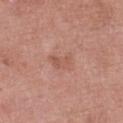notes — total-body-photography surveillance lesion; no biopsy | subject — female, roughly 70 years of age | image source — ~15 mm crop, total-body skin-cancer survey | automated metrics — a mean CIELAB color near L≈55 a*≈23 b*≈28, about 7 CIELAB-L* units darker than the surrounding skin, and a normalized border contrast of about 5 | lighting — white-light | lesion size — ≈3 mm | body site — the left thigh.